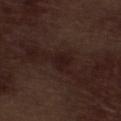<record>
  <biopsy_status>not biopsied; imaged during a skin examination</biopsy_status>
  <site>left lower leg</site>
  <automated_metrics>
    <shape_asymmetry>0.55</shape_asymmetry>
    <border_irregularity_0_10>6.5</border_irregularity_0_10>
    <color_variation_0_10>1.0</color_variation_0_10>
    <peripheral_color_asymmetry>0.5</peripheral_color_asymmetry>
    <nevus_likeness_0_100>0</nevus_likeness_0_100>
    <lesion_detection_confidence_0_100>100</lesion_detection_confidence_0_100>
  </automated_metrics>
  <image>
    <source>total-body photography crop</source>
    <field_of_view_mm>15</field_of_view_mm>
  </image>
  <patient>
    <sex>male</sex>
    <age_approx>70</age_approx>
  </patient>
  <lesion_size>
    <long_diameter_mm_approx>4.0</long_diameter_mm_approx>
  </lesion_size>
  <lighting>white-light</lighting>
</record>